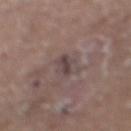| key | value |
|---|---|
| notes | catalogued during a skin exam; not biopsied |
| size | ~3 mm (longest diameter) |
| acquisition | ~15 mm tile from a whole-body skin photo |
| automated metrics | a lesion area of about 3 mm²; border irregularity of about 4.5 on a 0–10 scale and internal color variation of about 0 on a 0–10 scale; a nevus-likeness score of about 0/100 and lesion-presence confidence of about 55/100 |
| tile lighting | white-light illumination |
| patient | male, aged 63–67 |
| location | the right lower leg |